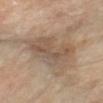Captured during whole-body skin photography for melanoma surveillance; the lesion was not biopsied. A 15 mm close-up extracted from a 3D total-body photography capture. Captured under cross-polarized illumination. The total-body-photography lesion software estimated a footprint of about 23 mm² and an outline eccentricity of about 0.9 (0 = round, 1 = elongated). And it measured a mean CIELAB color near L≈54 a*≈14 b*≈28. A female subject, about 55 years old. Measured at roughly 8.5 mm in maximum diameter. On the arm.